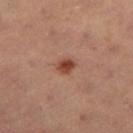Imaged during a routine full-body skin examination; the lesion was not biopsied and no histopathology is available. The lesion is located on the right thigh. This is a cross-polarized tile. Cropped from a total-body skin-imaging series; the visible field is about 15 mm. A female subject aged approximately 40. The recorded lesion diameter is about 2 mm.